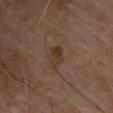Findings:
– follow-up · total-body-photography surveillance lesion; no biopsy
– image-analysis metrics · an outline eccentricity of about 0.65 (0 = round, 1 = elongated); an automated nevus-likeness rating near 45 out of 100 and a detector confidence of about 100 out of 100 that the crop contains a lesion
– tile lighting · cross-polarized
– diameter · ≈2.5 mm
– site · the upper back
– image source · ~15 mm tile from a whole-body skin photo
– patient · male, aged around 65